The lesion-visualizer software estimated a mean CIELAB color near L≈43 a*≈30 b*≈22, a lesion–skin lightness drop of about 5, and a normalized lesion–skin contrast near 4.5. And it measured a nevus-likeness score of about 0/100 and a detector confidence of about 45 out of 100 that the crop contains a lesion; a male subject aged around 45; approximately 1.5 mm at its widest; located on the head or neck; a 15 mm close-up extracted from a 3D total-body photography capture: 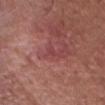diagnosis: a superficial basal cell carcinoma — a malignant skin lesion.